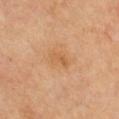follow-up: imaged on a skin check; not biopsied
lighting: cross-polarized illumination
subject: male, roughly 70 years of age
imaging modality: 15 mm crop, total-body photography
location: the chest
image-analysis metrics: a footprint of about 4 mm², an eccentricity of roughly 0.8, and two-axis asymmetry of about 0.3; an average lesion color of about L≈56 a*≈21 b*≈38 (CIELAB), a lesion–skin lightness drop of about 6, and a normalized border contrast of about 5.5; border irregularity of about 3 on a 0–10 scale, a color-variation rating of about 2.5/10, and radial color variation of about 1; a nevus-likeness score of about 0/100 and lesion-presence confidence of about 100/100
size: about 3 mm